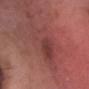* follow-up · no biopsy performed (imaged during a skin exam)
* lighting · white-light
* subject · male, approximately 50 years of age
* automated metrics · a mean CIELAB color near L≈42 a*≈26 b*≈23, about 6 CIELAB-L* units darker than the surrounding skin, and a normalized border contrast of about 5; a lesion-detection confidence of about 95/100
* imaging modality · ~15 mm crop, total-body skin-cancer survey
* body site · the head or neck
* lesion diameter · ~6.5 mm (longest diameter)